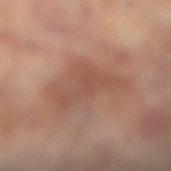Clinical impression:
The lesion was photographed on a routine skin check and not biopsied; there is no pathology result.
Image and clinical context:
The subject is a female approximately 80 years of age. The lesion's longest dimension is about 6.5 mm. Captured under cross-polarized illumination. Located on the left leg. This image is a 15 mm lesion crop taken from a total-body photograph.